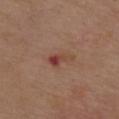| feature | finding |
|---|---|
| workup | no biopsy performed (imaged during a skin exam) |
| anatomic site | the upper back |
| lesion size | about 3 mm |
| image source | ~15 mm crop, total-body skin-cancer survey |
| tile lighting | white-light |
| patient | male, aged 68 to 72 |